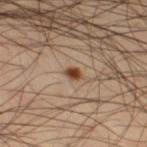Assessment: Recorded during total-body skin imaging; not selected for excision or biopsy. Acquisition and patient details: Imaged with cross-polarized lighting. The lesion is located on the leg. Automated tile analysis of the lesion measured an automated nevus-likeness rating near 100 out of 100. Cropped from a whole-body photographic skin survey; the tile spans about 15 mm. The subject is a male about 50 years old.